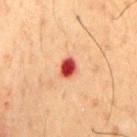Clinical impression: Part of a total-body skin-imaging series; this lesion was reviewed on a skin check and was not flagged for biopsy. Context: A male subject, in their 50s. On the front of the torso. Cropped from a total-body skin-imaging series; the visible field is about 15 mm. Imaged with cross-polarized lighting.